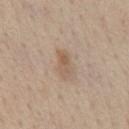  biopsy_status: not biopsied; imaged during a skin examination
  site: chest
  image:
    source: total-body photography crop
    field_of_view_mm: 15
  automated_metrics:
    cielab_L: 57
    cielab_a: 15
    cielab_b: 29
    vs_skin_darker_L: 8.0
    vs_skin_contrast_norm: 6.0
    nevus_likeness_0_100: 10
    lesion_detection_confidence_0_100: 100
  lighting: white-light
  patient:
    sex: male
    age_approx: 60